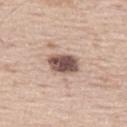A 15 mm close-up extracted from a 3D total-body photography capture.
An algorithmic analysis of the crop reported an average lesion color of about L≈52 a*≈17 b*≈22 (CIELAB), a lesion–skin lightness drop of about 19, and a normalized lesion–skin contrast near 12.5. The software also gave a border-irregularity index near 2/10, a color-variation rating of about 4.5/10, and a peripheral color-asymmetry measure near 1.5.
Imaged with white-light lighting.
A male subject, approximately 65 years of age.
The recorded lesion diameter is about 4 mm.
The lesion is located on the left thigh.